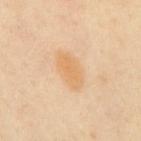Imaged during a routine full-body skin examination; the lesion was not biopsied and no histopathology is available. The lesion is on the mid back. This is a cross-polarized tile. A male patient, in their 50s. A lesion tile, about 15 mm wide, cut from a 3D total-body photograph. The recorded lesion diameter is about 5 mm.